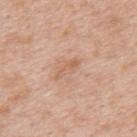No biopsy was performed on this lesion — it was imaged during a full skin examination and was not determined to be concerning. The total-body-photography lesion software estimated an area of roughly 4 mm² and a shape-asymmetry score of about 0.3 (0 = symmetric). The software also gave about 7 CIELAB-L* units darker than the surrounding skin and a normalized border contrast of about 5. The analysis additionally found a lesion-detection confidence of about 100/100. This is a white-light tile. Located on the upper back. A male patient aged approximately 50. A close-up tile cropped from a whole-body skin photograph, about 15 mm across.